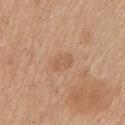Q: Was a biopsy performed?
A: no biopsy performed (imaged during a skin exam)
Q: What lighting was used for the tile?
A: white-light illumination
Q: How was this image acquired?
A: ~15 mm tile from a whole-body skin photo
Q: How large is the lesion?
A: about 2.5 mm
Q: Who is the patient?
A: female, aged approximately 65
Q: Where on the body is the lesion?
A: the upper back